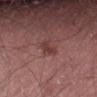No biopsy was performed on this lesion — it was imaged during a full skin examination and was not determined to be concerning. Cropped from a total-body skin-imaging series; the visible field is about 15 mm. About 2.5 mm across. A male patient, aged 48–52. The lesion is located on the lower back. Captured under white-light illumination.